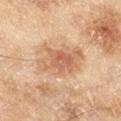Imaged during a routine full-body skin examination; the lesion was not biopsied and no histopathology is available. Approximately 6.5 mm at its widest. From the right lower leg. A region of skin cropped from a whole-body photographic capture, roughly 15 mm wide. The patient is a female approximately 60 years of age. Imaged with cross-polarized lighting.